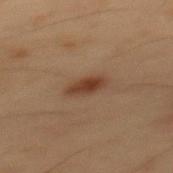Impression: No biopsy was performed on this lesion — it was imaged during a full skin examination and was not determined to be concerning. Background: Captured under cross-polarized illumination. A 15 mm close-up extracted from a 3D total-body photography capture. About 3.5 mm across. On the mid back. A male subject aged 53 to 57.